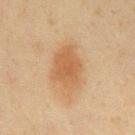Part of a total-body skin-imaging series; this lesion was reviewed on a skin check and was not flagged for biopsy.